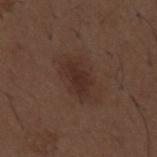Part of a total-body skin-imaging series; this lesion was reviewed on a skin check and was not flagged for biopsy.
Located on the mid back.
A region of skin cropped from a whole-body photographic capture, roughly 15 mm wide.
A male subject, approximately 50 years of age.
The total-body-photography lesion software estimated a lesion color around L≈29 a*≈17 b*≈22 in CIELAB and roughly 6 lightness units darker than nearby skin.
Imaged with white-light lighting.